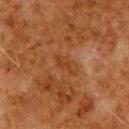biopsy_status: not biopsied; imaged during a skin examination
patient:
  sex: male
  age_approx: 80
lesion_size:
  long_diameter_mm_approx: 3.5
image:
  source: total-body photography crop
  field_of_view_mm: 15
automated_metrics:
  nevus_likeness_0_100: 0
site: upper back